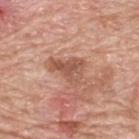This lesion was catalogued during total-body skin photography and was not selected for biopsy.
A 15 mm close-up extracted from a 3D total-body photography capture.
The lesion-visualizer software estimated a classifier nevus-likeness of about 0/100 and lesion-presence confidence of about 100/100.
From the upper back.
A male subject aged around 80.